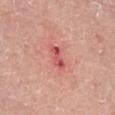biopsy_status: not biopsied; imaged during a skin examination
patient:
  sex: male
  age_approx: 65
lesion_size:
  long_diameter_mm_approx: 2.5
site: left lower leg
image:
  source: total-body photography crop
  field_of_view_mm: 15
automated_metrics:
  area_mm2_approx: 3.5
  eccentricity: 0.9
  shape_asymmetry: 0.25
  border_irregularity_0_10: 3.0
  color_variation_0_10: 3.0
  peripheral_color_asymmetry: 1.0
  nevus_likeness_0_100: 0
  lesion_detection_confidence_0_100: 100